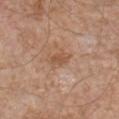biopsy_status: not biopsied; imaged during a skin examination
lighting: white-light
lesion_size:
  long_diameter_mm_approx: 3.0
image:
  source: total-body photography crop
  field_of_view_mm: 15
automated_metrics:
  area_mm2_approx: 4.0
  cielab_L: 53
  cielab_a: 20
  cielab_b: 32
  vs_skin_darker_L: 8.0
  vs_skin_contrast_norm: 6.0
  lesion_detection_confidence_0_100: 100
patient:
  sex: male
  age_approx: 65
site: front of the torso